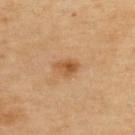{"biopsy_status": "not biopsied; imaged during a skin examination", "site": "upper back", "patient": {"sex": "female", "age_approx": 45}, "image": {"source": "total-body photography crop", "field_of_view_mm": 15}}